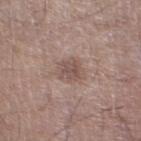| feature | finding |
|---|---|
| workup | no biopsy performed (imaged during a skin exam) |
| automated metrics | border irregularity of about 2.5 on a 0–10 scale and internal color variation of about 3 on a 0–10 scale |
| location | the left lower leg |
| subject | male, aged 58–62 |
| acquisition | ~15 mm tile from a whole-body skin photo |
| diameter | about 3 mm |